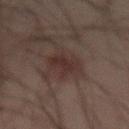Recorded during total-body skin imaging; not selected for excision or biopsy.
The subject is a male about 70 years old.
Cropped from a whole-body photographic skin survey; the tile spans about 15 mm.
The lesion's longest dimension is about 4.5 mm.
On the lower back.
Imaged with cross-polarized lighting.